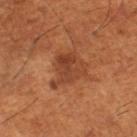notes: imaged on a skin check; not biopsied | subject: male, approximately 65 years of age | imaging modality: total-body-photography crop, ~15 mm field of view | tile lighting: cross-polarized | automated lesion analysis: an area of roughly 11 mm²; a lesion color around L≈43 a*≈27 b*≈35 in CIELAB, about 9 CIELAB-L* units darker than the surrounding skin, and a normalized lesion–skin contrast near 7; a nevus-likeness score of about 10/100 and a lesion-detection confidence of about 100/100 | location: the right lower leg.